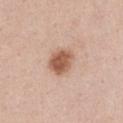Impression:
The lesion was photographed on a routine skin check and not biopsied; there is no pathology result.
Clinical summary:
From the chest. This image is a 15 mm lesion crop taken from a total-body photograph. Imaged with white-light lighting. The patient is a female about 40 years old. Longest diameter approximately 3.5 mm.